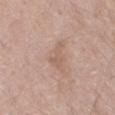Q: Is there a histopathology result?
A: no biopsy performed (imaged during a skin exam)
Q: What did automated image analysis measure?
A: an automated nevus-likeness rating near 0 out of 100 and a lesion-detection confidence of about 100/100
Q: Lesion location?
A: the left thigh
Q: How was the tile lit?
A: white-light illumination
Q: Lesion size?
A: about 3.5 mm
Q: Patient demographics?
A: male, aged 63–67
Q: What kind of image is this?
A: total-body-photography crop, ~15 mm field of view